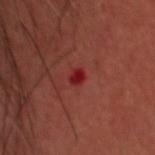No biopsy was performed on this lesion — it was imaged during a full skin examination and was not determined to be concerning.